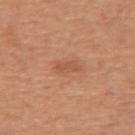Clinical impression: Captured during whole-body skin photography for melanoma surveillance; the lesion was not biopsied. Background: The patient is a female roughly 50 years of age. This image is a 15 mm lesion crop taken from a total-body photograph. Approximately 3.5 mm at its widest. The lesion is located on the arm. The total-body-photography lesion software estimated a lesion area of about 4 mm², an outline eccentricity of about 0.9 (0 = round, 1 = elongated), and a symmetry-axis asymmetry near 0.25. And it measured a lesion–skin lightness drop of about 7 and a lesion-to-skin contrast of about 5 (normalized; higher = more distinct). The analysis additionally found a peripheral color-asymmetry measure near 0.5.